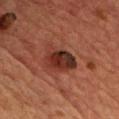notes: no biopsy performed (imaged during a skin exam) | patient: male, aged around 65 | size: ≈4 mm | image source: ~15 mm tile from a whole-body skin photo | illumination: cross-polarized illumination | location: the upper back.